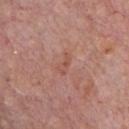Findings:
– notes · total-body-photography surveillance lesion; no biopsy
– image source · ~15 mm crop, total-body skin-cancer survey
– anatomic site · the front of the torso
– lesion size · ~2.5 mm (longest diameter)
– patient · male, aged 73 to 77
– tile lighting · white-light